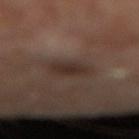Assessment:
The lesion was photographed on a routine skin check and not biopsied; there is no pathology result.
Clinical summary:
Captured under cross-polarized illumination. Located on the right lower leg. A 15 mm close-up extracted from a 3D total-body photography capture.